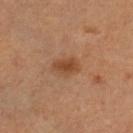biopsy status: no biopsy performed (imaged during a skin exam)
illumination: cross-polarized
patient: female, aged 58–62
image: ~15 mm tile from a whole-body skin photo
diameter: ≈3.5 mm
site: the left lower leg
automated metrics: an area of roughly 6 mm², a shape eccentricity near 0.75, and a shape-asymmetry score of about 0.25 (0 = symmetric); an average lesion color of about L≈48 a*≈22 b*≈35 (CIELAB), about 9 CIELAB-L* units darker than the surrounding skin, and a normalized lesion–skin contrast near 7.5; internal color variation of about 3.5 on a 0–10 scale and peripheral color asymmetry of about 1